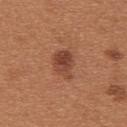follow-up: imaged on a skin check; not biopsied
lesion size: ~3.5 mm (longest diameter)
patient: female, aged around 30
anatomic site: the upper back
image source: ~15 mm tile from a whole-body skin photo